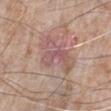Notes:
– workup · imaged on a skin check; not biopsied
– illumination · white-light illumination
– lesion diameter · ≈6 mm
– site · the left lower leg
– acquisition · ~15 mm crop, total-body skin-cancer survey
– subject · male, about 75 years old